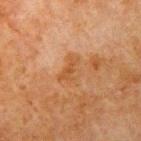Impression:
The lesion was tiled from a total-body skin photograph and was not biopsied.
Context:
Located on the left upper arm. The lesion's longest dimension is about 3 mm. A lesion tile, about 15 mm wide, cut from a 3D total-body photograph. This is a cross-polarized tile. A male subject roughly 80 years of age.